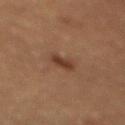Part of a total-body skin-imaging series; this lesion was reviewed on a skin check and was not flagged for biopsy.
On the mid back.
The subject is a female aged around 70.
An algorithmic analysis of the crop reported a mean CIELAB color near L≈31 a*≈17 b*≈25, a lesion–skin lightness drop of about 8, and a lesion-to-skin contrast of about 7.5 (normalized; higher = more distinct). And it measured a nevus-likeness score of about 90/100.
A lesion tile, about 15 mm wide, cut from a 3D total-body photograph.
Imaged with cross-polarized lighting.
Measured at roughly 2.5 mm in maximum diameter.